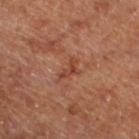workup: catalogued during a skin exam; not biopsied
imaging modality: total-body-photography crop, ~15 mm field of view
patient: male, roughly 60 years of age
lesion diameter: ~3 mm (longest diameter)
location: the left lower leg